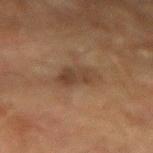  lighting: cross-polarized
  image:
    source: total-body photography crop
    field_of_view_mm: 15
  lesion_size:
    long_diameter_mm_approx: 4.5
  automated_metrics:
    vs_skin_darker_L: 7.0
    border_irregularity_0_10: 3.5
    color_variation_0_10: 3.5
    peripheral_color_asymmetry: 1.5
  site: right upper arm
  patient:
    sex: male
    age_approx: 75